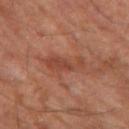The lesion is on the left thigh.
Automated image analysis of the tile measured an area of roughly 7.5 mm² and a shape eccentricity near 0.9. The analysis additionally found roughly 7 lightness units darker than nearby skin. It also reported a classifier nevus-likeness of about 0/100 and a detector confidence of about 100 out of 100 that the crop contains a lesion.
The subject is a male aged 68 to 72.
Cropped from a whole-body photographic skin survey; the tile spans about 15 mm.